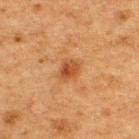Clinical impression:
No biopsy was performed on this lesion — it was imaged during a full skin examination and was not determined to be concerning.
Image and clinical context:
The lesion's longest dimension is about 2.5 mm. Imaged with cross-polarized lighting. An algorithmic analysis of the crop reported a lesion area of about 4.5 mm², an eccentricity of roughly 0.6, and two-axis asymmetry of about 0.2. The analysis additionally found a border-irregularity index near 2/10, a within-lesion color-variation index near 3.5/10, and a peripheral color-asymmetry measure near 1. A lesion tile, about 15 mm wide, cut from a 3D total-body photograph. The lesion is located on the upper back. The patient is a male in their mid- to late 70s.